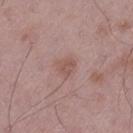– follow-up — no biopsy performed (imaged during a skin exam)
– subject — male, aged approximately 50
– image-analysis metrics — a shape eccentricity near 0.45 and a symmetry-axis asymmetry near 0.35
– lighting — white-light illumination
– anatomic site — the left thigh
– diameter — ~2.5 mm (longest diameter)
– acquisition — 15 mm crop, total-body photography